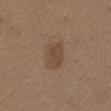The lesion was tiled from a total-body skin photograph and was not biopsied. A female subject about 40 years old. The recorded lesion diameter is about 3.5 mm. The lesion is on the abdomen. A 15 mm crop from a total-body photograph taken for skin-cancer surveillance. An algorithmic analysis of the crop reported an outline eccentricity of about 0.7 (0 = round, 1 = elongated) and a symmetry-axis asymmetry near 0.15. It also reported a lesion-detection confidence of about 100/100.